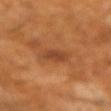Assessment:
No biopsy was performed on this lesion — it was imaged during a full skin examination and was not determined to be concerning.
Image and clinical context:
The patient is a male approximately 60 years of age. Automated image analysis of the tile measured a footprint of about 4.5 mm², an outline eccentricity of about 0.8 (0 = round, 1 = elongated), and two-axis asymmetry of about 0.2. The software also gave a mean CIELAB color near L≈39 a*≈24 b*≈33 and a lesion-to-skin contrast of about 6.5 (normalized; higher = more distinct). A 15 mm close-up tile from a total-body photography series done for melanoma screening. Measured at roughly 3 mm in maximum diameter. The lesion is located on the left forearm. This is a cross-polarized tile.